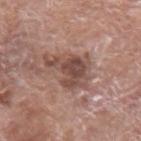Case summary:
- workup: total-body-photography surveillance lesion; no biopsy
- illumination: white-light
- patient: male, aged around 60
- TBP lesion metrics: an eccentricity of roughly 0.75 and a symmetry-axis asymmetry near 0.45; an average lesion color of about L≈48 a*≈20 b*≈23 (CIELAB), roughly 11 lightness units darker than nearby skin, and a lesion-to-skin contrast of about 8.5 (normalized; higher = more distinct); a border-irregularity index near 6/10 and internal color variation of about 4.5 on a 0–10 scale
- acquisition: ~15 mm tile from a whole-body skin photo
- anatomic site: the right upper arm